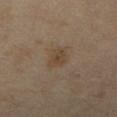The lesion was photographed on a routine skin check and not biopsied; there is no pathology result. The lesion-visualizer software estimated an eccentricity of roughly 0.6. The analysis additionally found a mean CIELAB color near L≈44 a*≈13 b*≈28, a lesion–skin lightness drop of about 6, and a lesion-to-skin contrast of about 6 (normalized; higher = more distinct). It also reported a border-irregularity rating of about 2/10, internal color variation of about 2 on a 0–10 scale, and a peripheral color-asymmetry measure near 0.5. About 3 mm across. A roughly 15 mm field-of-view crop from a total-body skin photograph. The tile uses cross-polarized illumination. A female subject, aged 68–72. On the leg.